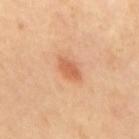Imaged during a routine full-body skin examination; the lesion was not biopsied and no histopathology is available. From the back. This image is a 15 mm lesion crop taken from a total-body photograph.